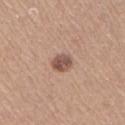No biopsy was performed on this lesion — it was imaged during a full skin examination and was not determined to be concerning. A female patient about 65 years old. From the right upper arm. Captured under white-light illumination. A 15 mm close-up extracted from a 3D total-body photography capture.